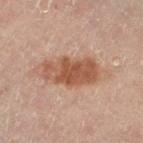  biopsy_status: not biopsied; imaged during a skin examination
  patient:
    sex: female
    age_approx: 60
  image:
    source: total-body photography crop
    field_of_view_mm: 15
  automated_metrics:
    area_mm2_approx: 15.0
    eccentricity: 0.85
    shape_asymmetry: 0.2
    border_irregularity_0_10: 2.5
    color_variation_0_10: 4.5
    peripheral_color_asymmetry: 1.5
    nevus_likeness_0_100: 85
  lighting: cross-polarized
  site: left thigh